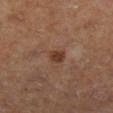Q: Was a biopsy performed?
A: total-body-photography surveillance lesion; no biopsy
Q: Lesion size?
A: ≈2 mm
Q: What lighting was used for the tile?
A: cross-polarized
Q: How was this image acquired?
A: ~15 mm crop, total-body skin-cancer survey
Q: Lesion location?
A: the left lower leg
Q: Who is the patient?
A: female, in their 40s